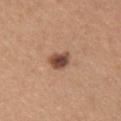Q: Was this lesion biopsied?
A: no biopsy performed (imaged during a skin exam)
Q: How was this image acquired?
A: 15 mm crop, total-body photography
Q: What lighting was used for the tile?
A: white-light illumination
Q: What is the anatomic site?
A: the front of the torso
Q: What are the patient's age and sex?
A: female, in their 40s
Q: Automated lesion metrics?
A: a nevus-likeness score of about 95/100 and a detector confidence of about 100 out of 100 that the crop contains a lesion